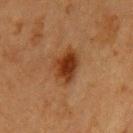biopsy status=imaged on a skin check; not biopsied | body site=the arm | subject=female, aged approximately 55 | diameter=~4 mm (longest diameter) | image=~15 mm crop, total-body skin-cancer survey.